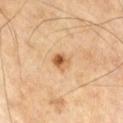acquisition = 15 mm crop, total-body photography | diameter = about 2.5 mm | tile lighting = cross-polarized | body site = the right thigh | automated metrics = a border-irregularity rating of about 2/10 and peripheral color asymmetry of about 3 | patient = male, aged approximately 70.